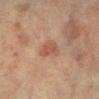follow-up: no biopsy performed (imaged during a skin exam)
size: ≈2.5 mm
subject: female, in their 70s
anatomic site: the right lower leg
automated lesion analysis: an area of roughly 4 mm², a shape eccentricity near 0.8, and a shape-asymmetry score of about 0.25 (0 = symmetric); about 8 CIELAB-L* units darker than the surrounding skin and a lesion-to-skin contrast of about 6.5 (normalized; higher = more distinct); lesion-presence confidence of about 100/100
acquisition: 15 mm crop, total-body photography
tile lighting: cross-polarized